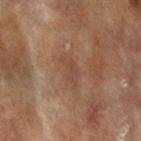follow-up: catalogued during a skin exam; not biopsied
patient: female, roughly 75 years of age
image source: total-body-photography crop, ~15 mm field of view
lesion diameter: about 4 mm
automated lesion analysis: a footprint of about 5 mm², a shape eccentricity near 0.9, and a shape-asymmetry score of about 0.4 (0 = symmetric); a border-irregularity index near 4.5/10, a color-variation rating of about 1/10, and radial color variation of about 0.5; a nevus-likeness score of about 0/100 and a detector confidence of about 95 out of 100 that the crop contains a lesion
site: the left forearm
tile lighting: cross-polarized illumination